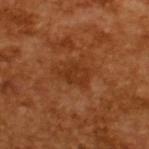biopsy status — catalogued during a skin exam; not biopsied | lesion size — ≈3.5 mm | tile lighting — cross-polarized | acquisition — 15 mm crop, total-body photography | automated lesion analysis — an eccentricity of roughly 0.75 and a symmetry-axis asymmetry near 0.35; a border-irregularity index near 4/10 and peripheral color asymmetry of about 0.5 | subject — male, aged approximately 65.